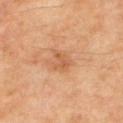| key | value |
|---|---|
| notes | no biopsy performed (imaged during a skin exam) |
| illumination | cross-polarized illumination |
| patient | male, aged 53–57 |
| site | the mid back |
| image source | 15 mm crop, total-body photography |